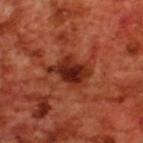Part of a total-body skin-imaging series; this lesion was reviewed on a skin check and was not flagged for biopsy. A close-up tile cropped from a whole-body skin photograph, about 15 mm across. Located on the upper back. Longest diameter approximately 5 mm. A male subject approximately 70 years of age. Automated image analysis of the tile measured a mean CIELAB color near L≈30 a*≈30 b*≈31 and about 11 CIELAB-L* units darker than the surrounding skin. The analysis additionally found border irregularity of about 4.5 on a 0–10 scale and a color-variation rating of about 6/10.